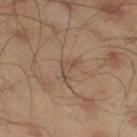Recorded during total-body skin imaging; not selected for excision or biopsy. On the leg. A 15 mm close-up extracted from a 3D total-body photography capture. A male subject, in their mid-40s.